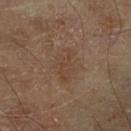Q: Was this lesion biopsied?
A: catalogued during a skin exam; not biopsied
Q: What is the imaging modality?
A: total-body-photography crop, ~15 mm field of view
Q: What did automated image analysis measure?
A: border irregularity of about 3.5 on a 0–10 scale, a color-variation rating of about 2/10, and a peripheral color-asymmetry measure near 0.5
Q: Lesion location?
A: the right lower leg
Q: What are the patient's age and sex?
A: male, aged 68–72
Q: How was the tile lit?
A: cross-polarized
Q: How large is the lesion?
A: about 4 mm